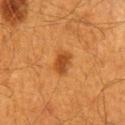Recorded during total-body skin imaging; not selected for excision or biopsy. The lesion is located on the mid back. This image is a 15 mm lesion crop taken from a total-body photograph. The total-body-photography lesion software estimated an area of roughly 5 mm², a shape eccentricity near 0.7, and two-axis asymmetry of about 0.15. And it measured a nevus-likeness score of about 95/100. A male subject, aged around 60. This is a cross-polarized tile. Longest diameter approximately 3 mm.